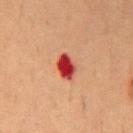<case>
  <patient>
    <sex>male</sex>
    <age_approx>55</age_approx>
  </patient>
  <image>
    <source>total-body photography crop</source>
    <field_of_view_mm>15</field_of_view_mm>
  </image>
  <lesion_size>
    <long_diameter_mm_approx>3.5</long_diameter_mm_approx>
  </lesion_size>
  <site>back</site>
  <lighting>cross-polarized</lighting>
</case>